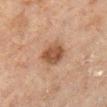Findings:
* biopsy status: no biopsy performed (imaged during a skin exam)
* body site: the right lower leg
* image: ~15 mm tile from a whole-body skin photo
* patient: male, aged 68–72
* tile lighting: cross-polarized